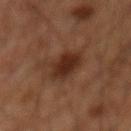Clinical impression: The lesion was photographed on a routine skin check and not biopsied; there is no pathology result. Image and clinical context: The tile uses cross-polarized illumination. A male subject, about 60 years old. The lesion-visualizer software estimated a mean CIELAB color near L≈25 a*≈19 b*≈25, a lesion–skin lightness drop of about 9, and a normalized lesion–skin contrast near 9.5. On the mid back. A roughly 15 mm field-of-view crop from a total-body skin photograph.